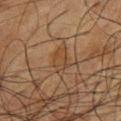This lesion was catalogued during total-body skin photography and was not selected for biopsy.
A male subject, aged approximately 60.
Located on the chest.
This image is a 15 mm lesion crop taken from a total-body photograph.